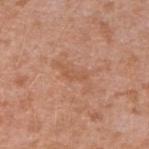{"biopsy_status": "not biopsied; imaged during a skin examination", "lighting": "white-light", "patient": {"sex": "female", "age_approx": 40}, "lesion_size": {"long_diameter_mm_approx": 3.0}, "image": {"source": "total-body photography crop", "field_of_view_mm": 15}, "site": "right forearm"}